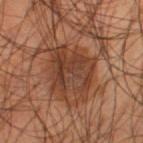Impression: The lesion was photographed on a routine skin check and not biopsied; there is no pathology result. Context: Longest diameter approximately 6 mm. A 15 mm close-up extracted from a 3D total-body photography capture. On the left thigh. The subject is a male in their mid-50s.